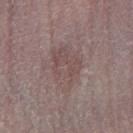The lesion was photographed on a routine skin check and not biopsied; there is no pathology result. About 5.5 mm across. Automated tile analysis of the lesion measured an average lesion color of about L≈48 a*≈16 b*≈17 (CIELAB), about 6 CIELAB-L* units darker than the surrounding skin, and a normalized border contrast of about 5. The lesion is on the right lower leg. A male subject, aged 73–77. This image is a 15 mm lesion crop taken from a total-body photograph. This is a white-light tile.